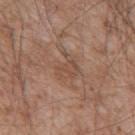Part of a total-body skin-imaging series; this lesion was reviewed on a skin check and was not flagged for biopsy.
Located on the mid back.
This image is a 15 mm lesion crop taken from a total-body photograph.
The tile uses white-light illumination.
A male patient roughly 55 years of age.